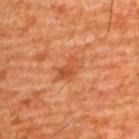Recorded during total-body skin imaging; not selected for excision or biopsy.